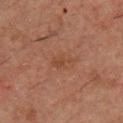Q: Was this lesion biopsied?
A: catalogued during a skin exam; not biopsied
Q: What is the imaging modality?
A: ~15 mm tile from a whole-body skin photo
Q: Automated lesion metrics?
A: a lesion color around L≈34 a*≈18 b*≈26 in CIELAB, roughly 4 lightness units darker than nearby skin, and a lesion-to-skin contrast of about 5.5 (normalized; higher = more distinct); internal color variation of about 1 on a 0–10 scale
Q: What lighting was used for the tile?
A: cross-polarized illumination
Q: What is the anatomic site?
A: the chest
Q: Patient demographics?
A: male, in their 50s
Q: Lesion size?
A: ≈3 mm